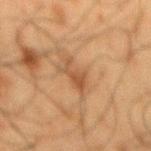Q: Is there a histopathology result?
A: total-body-photography surveillance lesion; no biopsy
Q: What is the imaging modality?
A: 15 mm crop, total-body photography
Q: What are the patient's age and sex?
A: male, aged around 60
Q: Where on the body is the lesion?
A: the mid back